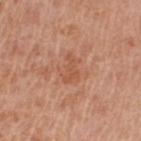| key | value |
|---|---|
| notes | catalogued during a skin exam; not biopsied |
| imaging modality | 15 mm crop, total-body photography |
| body site | the arm |
| patient | female, aged around 55 |